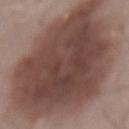The lesion's longest dimension is about 17 mm. Automated tile analysis of the lesion measured a border-irregularity index near 3/10, internal color variation of about 5.5 on a 0–10 scale, and a peripheral color-asymmetry measure near 2. The analysis additionally found a classifier nevus-likeness of about 80/100 and a detector confidence of about 100 out of 100 that the crop contains a lesion. Located on the mid back. The tile uses white-light illumination. A close-up tile cropped from a whole-body skin photograph, about 15 mm across. A male patient, roughly 55 years of age.